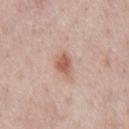Impression:
The lesion was tiled from a total-body skin photograph and was not biopsied.
Context:
The lesion-visualizer software estimated border irregularity of about 3.5 on a 0–10 scale, a within-lesion color-variation index near 2.5/10, and peripheral color asymmetry of about 1. The software also gave a nevus-likeness score of about 90/100 and a detector confidence of about 100 out of 100 that the crop contains a lesion. Captured under white-light illumination. The lesion is on the chest. A male subject, aged around 50. A 15 mm crop from a total-body photograph taken for skin-cancer surveillance. Measured at roughly 3 mm in maximum diameter.